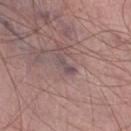Captured during whole-body skin photography for melanoma surveillance; the lesion was not biopsied.
Automated tile analysis of the lesion measured an eccentricity of roughly 0.95 and a symmetry-axis asymmetry near 0.35. The analysis additionally found border irregularity of about 4.5 on a 0–10 scale.
A region of skin cropped from a whole-body photographic capture, roughly 15 mm wide.
This is a white-light tile.
From the right lower leg.
A male patient, aged approximately 65.
Measured at roughly 2.5 mm in maximum diameter.